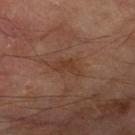follow-up: imaged on a skin check; not biopsied | lesion diameter: ~3.5 mm (longest diameter) | TBP lesion metrics: a classifier nevus-likeness of about 0/100 and lesion-presence confidence of about 100/100 | illumination: cross-polarized | patient: male, aged 68–72 | image: ~15 mm crop, total-body skin-cancer survey | body site: the right thigh.